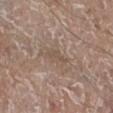Impression:
Captured during whole-body skin photography for melanoma surveillance; the lesion was not biopsied.
Acquisition and patient details:
The lesion-visualizer software estimated an eccentricity of roughly 0.85 and a symmetry-axis asymmetry near 0.35. It also reported roughly 6 lightness units darker than nearby skin. And it measured a border-irregularity rating of about 3.5/10 and a within-lesion color-variation index near 0/10. This is a white-light tile. Approximately 3 mm at its widest. Cropped from a whole-body photographic skin survey; the tile spans about 15 mm. A male subject roughly 80 years of age. From the right lower leg.